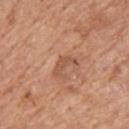{"biopsy_status": "not biopsied; imaged during a skin examination", "lesion_size": {"long_diameter_mm_approx": 3.5}, "site": "upper back", "image": {"source": "total-body photography crop", "field_of_view_mm": 15}, "automated_metrics": {"area_mm2_approx": 3.5, "eccentricity": 0.9, "shape_asymmetry": 0.55, "border_irregularity_0_10": 6.0, "color_variation_0_10": 0.5, "lesion_detection_confidence_0_100": 100}, "patient": {"sex": "male", "age_approx": 60}, "lighting": "white-light"}